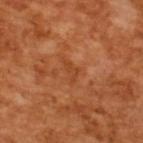Assessment: Captured during whole-body skin photography for melanoma surveillance; the lesion was not biopsied. Clinical summary: A male patient, aged 63 to 67. This is a cross-polarized tile. Approximately 2.5 mm at its widest. A 15 mm crop from a total-body photograph taken for skin-cancer surveillance.